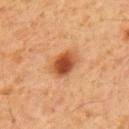Clinical impression:
Part of a total-body skin-imaging series; this lesion was reviewed on a skin check and was not flagged for biopsy.
Acquisition and patient details:
A lesion tile, about 15 mm wide, cut from a 3D total-body photograph. The tile uses cross-polarized illumination. On the upper back. About 3.5 mm across. A male subject roughly 60 years of age.